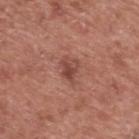Assessment: This lesion was catalogued during total-body skin photography and was not selected for biopsy. Acquisition and patient details: Imaged with white-light lighting. A male patient, roughly 75 years of age. From the upper back. A roughly 15 mm field-of-view crop from a total-body skin photograph.